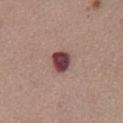Recorded during total-body skin imaging; not selected for excision or biopsy.
The patient is a female aged 33 to 37.
On the chest.
The lesion-visualizer software estimated an area of roughly 6.5 mm², a shape eccentricity near 0.5, and two-axis asymmetry of about 0.2. And it measured a border-irregularity index near 2/10 and peripheral color asymmetry of about 1.5.
The lesion's longest dimension is about 3 mm.
A 15 mm crop from a total-body photograph taken for skin-cancer surveillance.
Captured under white-light illumination.